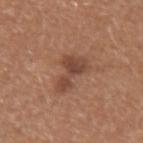The lesion was tiled from a total-body skin photograph and was not biopsied. From the right upper arm. Automated tile analysis of the lesion measured an area of roughly 8 mm², an eccentricity of roughly 0.85, and two-axis asymmetry of about 0.6. The software also gave a border-irregularity rating of about 7/10, a within-lesion color-variation index near 3.5/10, and peripheral color asymmetry of about 1. A 15 mm close-up extracted from a 3D total-body photography capture. The subject is a female approximately 35 years of age.